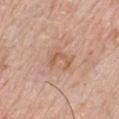No biopsy was performed on this lesion — it was imaged during a full skin examination and was not determined to be concerning. The lesion is located on the chest. A male subject, aged 78–82. Cropped from a total-body skin-imaging series; the visible field is about 15 mm. An algorithmic analysis of the crop reported a border-irregularity index near 7.5/10, a within-lesion color-variation index near 1/10, and a peripheral color-asymmetry measure near 0.5. The software also gave an automated nevus-likeness rating near 0 out of 100.